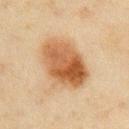Imaged during a routine full-body skin examination; the lesion was not biopsied and no histopathology is available.